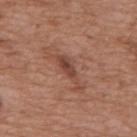follow-up: total-body-photography surveillance lesion; no biopsy
diameter: about 5 mm
tile lighting: white-light
location: the back
automated metrics: an average lesion color of about L≈45 a*≈22 b*≈27 (CIELAB), about 9 CIELAB-L* units darker than the surrounding skin, and a normalized lesion–skin contrast near 7
patient: female, aged 73 to 77
image: 15 mm crop, total-body photography